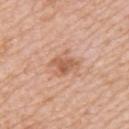Q: Lesion size?
A: ~3.5 mm (longest diameter)
Q: Who is the patient?
A: female, in their mid- to late 40s
Q: How was the tile lit?
A: white-light illumination
Q: Lesion location?
A: the upper back
Q: What is the imaging modality?
A: ~15 mm crop, total-body skin-cancer survey